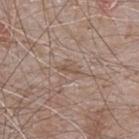follow-up: total-body-photography surveillance lesion; no biopsy | patient: male, in their mid-60s | acquisition: 15 mm crop, total-body photography | anatomic site: the chest | automated lesion analysis: an area of roughly 2.5 mm², an outline eccentricity of about 0.85 (0 = round, 1 = elongated), and two-axis asymmetry of about 0.4; a mean CIELAB color near L≈52 a*≈15 b*≈26, roughly 7 lightness units darker than nearby skin, and a normalized border contrast of about 6; a border-irregularity rating of about 4/10 and peripheral color asymmetry of about 0; an automated nevus-likeness rating near 0 out of 100 and a detector confidence of about 85 out of 100 that the crop contains a lesion | lesion diameter: ~2.5 mm (longest diameter).